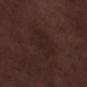{"biopsy_status": "not biopsied; imaged during a skin examination", "image": {"source": "total-body photography crop", "field_of_view_mm": 15}, "automated_metrics": {"area_mm2_approx": 11.0, "border_irregularity_0_10": 3.0, "color_variation_0_10": 2.5}, "lighting": "white-light", "patient": {"sex": "male", "age_approx": 70}, "site": "leg"}